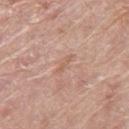The lesion was tiled from a total-body skin photograph and was not biopsied.
From the right thigh.
The lesion-visualizer software estimated a footprint of about 2 mm² and two-axis asymmetry of about 0.35. The analysis additionally found an automated nevus-likeness rating near 0 out of 100.
Cropped from a total-body skin-imaging series; the visible field is about 15 mm.
Measured at roughly 2.5 mm in maximum diameter.
Captured under white-light illumination.
A female subject, approximately 60 years of age.